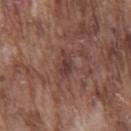Clinical impression:
Captured during whole-body skin photography for melanoma surveillance; the lesion was not biopsied.